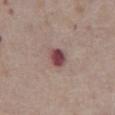{"biopsy_status": "not biopsied; imaged during a skin examination", "automated_metrics": {"cielab_L": 46, "cielab_a": 23, "cielab_b": 17, "vs_skin_darker_L": 14.0, "vs_skin_contrast_norm": 10.5, "color_variation_0_10": 4.5, "peripheral_color_asymmetry": 1.5, "nevus_likeness_0_100": 0, "lesion_detection_confidence_0_100": 100}, "image": {"source": "total-body photography crop", "field_of_view_mm": 15}, "lesion_size": {"long_diameter_mm_approx": 2.5}, "site": "abdomen", "patient": {"sex": "male", "age_approx": 75}}